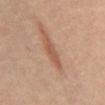{
  "biopsy_status": "not biopsied; imaged during a skin examination"
}